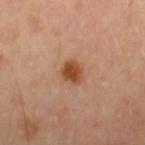{"biopsy_status": "not biopsied; imaged during a skin examination", "patient": {"sex": "female", "age_approx": 55}, "image": {"source": "total-body photography crop", "field_of_view_mm": 15}, "lighting": "cross-polarized", "site": "left forearm", "automated_metrics": {"cielab_L": 50, "cielab_a": 25, "cielab_b": 36, "vs_skin_darker_L": 12.0, "vs_skin_contrast_norm": 9.5, "border_irregularity_0_10": 1.5, "color_variation_0_10": 3.5, "nevus_likeness_0_100": 100, "lesion_detection_confidence_0_100": 100}}